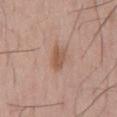No biopsy was performed on this lesion — it was imaged during a full skin examination and was not determined to be concerning. A lesion tile, about 15 mm wide, cut from a 3D total-body photograph. A male patient aged 53 to 57. From the mid back. Automated tile analysis of the lesion measured a lesion color around L≈55 a*≈19 b*≈29 in CIELAB, about 9 CIELAB-L* units darker than the surrounding skin, and a normalized lesion–skin contrast near 7. The recorded lesion diameter is about 3 mm. Imaged with white-light lighting.